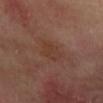| key | value |
|---|---|
| biopsy status | imaged on a skin check; not biopsied |
| anatomic site | the right forearm |
| automated lesion analysis | an area of roughly 6 mm²; a border-irregularity rating of about 2.5/10, a color-variation rating of about 2.5/10, and a peripheral color-asymmetry measure near 1 |
| patient | female, about 55 years old |
| image source | 15 mm crop, total-body photography |